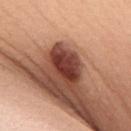follow-up: total-body-photography surveillance lesion; no biopsy | subject: male, about 40 years old | image source: 15 mm crop, total-body photography | body site: the upper back | tile lighting: white-light illumination | lesion diameter: ~4.5 mm (longest diameter).